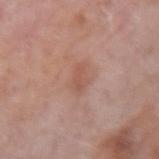Captured during whole-body skin photography for melanoma surveillance; the lesion was not biopsied.
A female patient, approximately 65 years of age.
The total-body-photography lesion software estimated a mean CIELAB color near L≈54 a*≈22 b*≈28, roughly 7 lightness units darker than nearby skin, and a normalized lesion–skin contrast near 5.5. And it measured border irregularity of about 3.5 on a 0–10 scale, internal color variation of about 0.5 on a 0–10 scale, and radial color variation of about 0.
This image is a 15 mm lesion crop taken from a total-body photograph.
This is a white-light tile.
About 2.5 mm across.
Located on the right forearm.